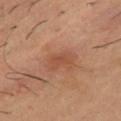{
  "biopsy_status": "not biopsied; imaged during a skin examination",
  "lighting": "cross-polarized",
  "patient": {
    "sex": "male",
    "age_approx": 40
  },
  "image": {
    "source": "total-body photography crop",
    "field_of_view_mm": 15
  },
  "site": "chest",
  "lesion_size": {
    "long_diameter_mm_approx": 3.0
  }
}